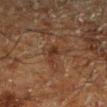| key | value |
|---|---|
| notes | catalogued during a skin exam; not biopsied |
| site | the left lower leg |
| automated metrics | about 6 CIELAB-L* units darker than the surrounding skin and a normalized lesion–skin contrast near 7.5; a border-irregularity rating of about 4/10, internal color variation of about 0.5 on a 0–10 scale, and peripheral color asymmetry of about 0; a nevus-likeness score of about 5/100 and a lesion-detection confidence of about 100/100 |
| imaging modality | total-body-photography crop, ~15 mm field of view |
| subject | male, in their 60s |
| lesion diameter | ~2.5 mm (longest diameter) |